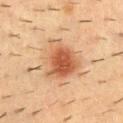A male patient aged 53 to 57. Automated tile analysis of the lesion measured a mean CIELAB color near L≈50 a*≈22 b*≈33, about 12 CIELAB-L* units darker than the surrounding skin, and a normalized lesion–skin contrast near 8.5. The software also gave a classifier nevus-likeness of about 100/100. Cropped from a whole-body photographic skin survey; the tile spans about 15 mm. This is a cross-polarized tile. On the mid back.